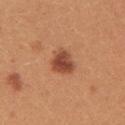{"image": {"source": "total-body photography crop", "field_of_view_mm": 15}, "site": "right upper arm", "lighting": "white-light", "automated_metrics": {"border_irregularity_0_10": 2.5, "color_variation_0_10": 3.5, "peripheral_color_asymmetry": 1.0, "nevus_likeness_0_100": 100, "lesion_detection_confidence_0_100": 100}, "patient": {"sex": "female", "age_approx": 25}}